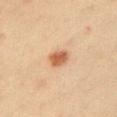Q: Is there a histopathology result?
A: no biopsy performed (imaged during a skin exam)
Q: What kind of image is this?
A: ~15 mm crop, total-body skin-cancer survey
Q: Who is the patient?
A: male, aged approximately 40
Q: Lesion location?
A: the left upper arm
Q: Automated lesion metrics?
A: a lesion area of about 5 mm², a shape eccentricity near 0.65, and two-axis asymmetry of about 0.2; a border-irregularity rating of about 1.5/10, internal color variation of about 2 on a 0–10 scale, and radial color variation of about 0.5